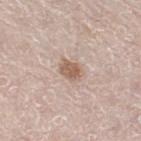No biopsy was performed on this lesion — it was imaged during a full skin examination and was not determined to be concerning.
Measured at roughly 3 mm in maximum diameter.
A close-up tile cropped from a whole-body skin photograph, about 15 mm across.
A female subject approximately 45 years of age.
From the right thigh.
The tile uses white-light illumination.